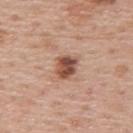{"biopsy_status": "not biopsied; imaged during a skin examination", "lighting": "white-light", "image": {"source": "total-body photography crop", "field_of_view_mm": 15}, "patient": {"sex": "male", "age_approx": 60}, "site": "upper back"}